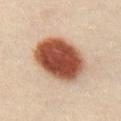<lesion>
<biopsy_status>not biopsied; imaged during a skin examination</biopsy_status>
</lesion>